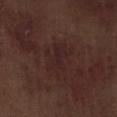Case summary:
* notes: catalogued during a skin exam; not biopsied
* automated metrics: a lesion area of about 9.5 mm², an eccentricity of roughly 0.75, and two-axis asymmetry of about 0.45; a border-irregularity rating of about 6.5/10 and radial color variation of about 0.5
* patient: male, aged approximately 70
* illumination: white-light illumination
* lesion diameter: about 4.5 mm
* site: the right lower leg
* image source: ~15 mm crop, total-body skin-cancer survey